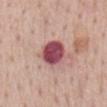Case summary:
- biopsy status — no biopsy performed (imaged during a skin exam)
- patient — female, in their mid-60s
- tile lighting — white-light illumination
- site — the mid back
- lesion size — ~4 mm (longest diameter)
- automated lesion analysis — an outline eccentricity of about 0.5 (0 = round, 1 = elongated) and a shape-asymmetry score of about 0.15 (0 = symmetric)
- image — ~15 mm crop, total-body skin-cancer survey